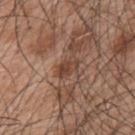Part of a total-body skin-imaging series; this lesion was reviewed on a skin check and was not flagged for biopsy. From the upper back. Approximately 3 mm at its widest. The tile uses white-light illumination. A male patient, aged approximately 50. Cropped from a total-body skin-imaging series; the visible field is about 15 mm.